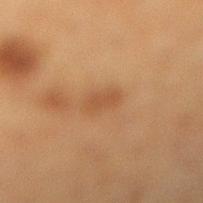  biopsy_status: not biopsied; imaged during a skin examination
  image:
    source: total-body photography crop
    field_of_view_mm: 15
  site: leg
  patient:
    sex: female
    age_approx: 40